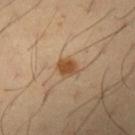workup: no biopsy performed (imaged during a skin exam)
tile lighting: cross-polarized
body site: the left upper arm
lesion diameter: ≈2.5 mm
subject: male, aged 38–42
image: 15 mm crop, total-body photography